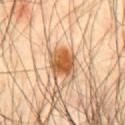Imaged during a routine full-body skin examination; the lesion was not biopsied and no histopathology is available. Captured under cross-polarized illumination. A region of skin cropped from a whole-body photographic capture, roughly 15 mm wide. A male subject aged 43 to 47. From the chest. The lesion's longest dimension is about 3.5 mm.